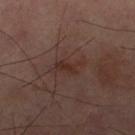{"biopsy_status": "not biopsied; imaged during a skin examination", "site": "right thigh", "automated_metrics": {"area_mm2_approx": 4.0, "eccentricity": 0.85, "shape_asymmetry": 0.4, "cielab_L": 30, "cielab_a": 19, "cielab_b": 22, "vs_skin_darker_L": 6.0, "vs_skin_contrast_norm": 6.5}, "image": {"source": "total-body photography crop", "field_of_view_mm": 15}}